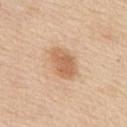This lesion was catalogued during total-body skin photography and was not selected for biopsy.